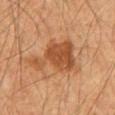Q: Was this lesion biopsied?
A: total-body-photography surveillance lesion; no biopsy
Q: How was this image acquired?
A: ~15 mm crop, total-body skin-cancer survey
Q: Lesion location?
A: the mid back
Q: Patient demographics?
A: male, aged approximately 60